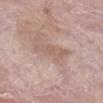Impression: The lesion was tiled from a total-body skin photograph and was not biopsied.